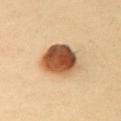Imaged during a routine full-body skin examination; the lesion was not biopsied and no histopathology is available.
Located on the chest.
About 4.5 mm across.
Automated tile analysis of the lesion measured a footprint of about 15 mm², a shape eccentricity near 0.35, and a symmetry-axis asymmetry near 0.15. The software also gave a lesion–skin lightness drop of about 23 and a normalized lesion–skin contrast near 14. And it measured a border-irregularity index near 1.5/10 and a within-lesion color-variation index near 8.5/10. The software also gave a classifier nevus-likeness of about 100/100 and lesion-presence confidence of about 100/100.
The patient is a female in their mid- to late 30s.
A region of skin cropped from a whole-body photographic capture, roughly 15 mm wide.